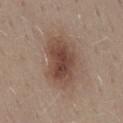body site: the lower back
image: ~15 mm tile from a whole-body skin photo
lighting: white-light
patient: female, aged around 45